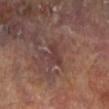Clinical impression:
The lesion was photographed on a routine skin check and not biopsied; there is no pathology result.
Clinical summary:
A region of skin cropped from a whole-body photographic capture, roughly 15 mm wide. Located on the right lower leg. The lesion's longest dimension is about 2.5 mm. This is a cross-polarized tile. A female subject, aged 73–77.